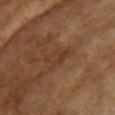No biopsy was performed on this lesion — it was imaged during a full skin examination and was not determined to be concerning. The recorded lesion diameter is about 3.5 mm. Automated image analysis of the tile measured a classifier nevus-likeness of about 0/100 and lesion-presence confidence of about 85/100. Cropped from a whole-body photographic skin survey; the tile spans about 15 mm. A female subject, about 75 years old. The lesion is located on the front of the torso.